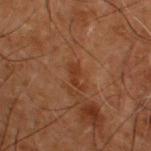workup — no biopsy performed (imaged during a skin exam)
anatomic site — the upper back
lighting — cross-polarized
subject — male, in their 50s
lesion diameter — ~2.5 mm (longest diameter)
image source — total-body-photography crop, ~15 mm field of view